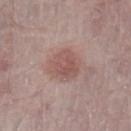Q: Was this lesion biopsied?
A: no biopsy performed (imaged during a skin exam)
Q: What is the anatomic site?
A: the left lower leg
Q: What kind of image is this?
A: ~15 mm tile from a whole-body skin photo
Q: Automated lesion metrics?
A: an area of roughly 10 mm², a shape eccentricity near 0.3, and two-axis asymmetry of about 0.25; roughly 8 lightness units darker than nearby skin and a lesion-to-skin contrast of about 6 (normalized; higher = more distinct)
Q: Illumination type?
A: white-light
Q: What is the lesion's diameter?
A: about 3.5 mm
Q: Who is the patient?
A: female, aged approximately 65